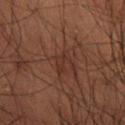Captured during whole-body skin photography for melanoma surveillance; the lesion was not biopsied.
Located on the right thigh.
A 15 mm close-up extracted from a 3D total-body photography capture.
The patient is a male aged around 50.